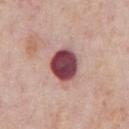<lesion>
  <biopsy_status>not biopsied; imaged during a skin examination</biopsy_status>
  <patient>
    <sex>male</sex>
    <age_approx>75</age_approx>
  </patient>
  <site>chest</site>
  <image>
    <source>total-body photography crop</source>
    <field_of_view_mm>15</field_of_view_mm>
  </image>
</lesion>